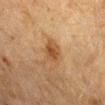The lesion was photographed on a routine skin check and not biopsied; there is no pathology result. A roughly 15 mm field-of-view crop from a total-body skin photograph. On the left forearm. About 2.5 mm across. The subject is a female roughly 55 years of age. Captured under cross-polarized illumination.